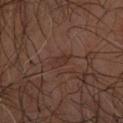<tbp_lesion>
  <biopsy_status>not biopsied; imaged during a skin examination</biopsy_status>
  <image>
    <source>total-body photography crop</source>
    <field_of_view_mm>15</field_of_view_mm>
  </image>
  <patient>
    <sex>male</sex>
    <age_approx>65</age_approx>
  </patient>
  <lighting>cross-polarized</lighting>
  <site>left forearm</site>
  <lesion_size>
    <long_diameter_mm_approx>2.5</long_diameter_mm_approx>
  </lesion_size>
</tbp_lesion>